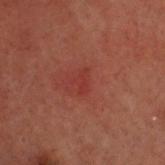This lesion was catalogued during total-body skin photography and was not selected for biopsy. Captured under cross-polarized illumination. The recorded lesion diameter is about 2.5 mm. Located on the head or neck. Cropped from a total-body skin-imaging series; the visible field is about 15 mm. A male subject about 50 years old. Automated image analysis of the tile measured a footprint of about 2.5 mm², an eccentricity of roughly 0.9, and a symmetry-axis asymmetry near 0.55. It also reported roughly 4 lightness units darker than nearby skin and a normalized border contrast of about 4.5. The software also gave a border-irregularity index near 5/10, a color-variation rating of about 0/10, and a peripheral color-asymmetry measure near 0. And it measured a classifier nevus-likeness of about 5/100.